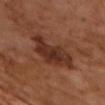Captured during whole-body skin photography for melanoma surveillance; the lesion was not biopsied.
The tile uses cross-polarized illumination.
The lesion's longest dimension is about 5.5 mm.
A close-up tile cropped from a whole-body skin photograph, about 15 mm across.
The lesion-visualizer software estimated an average lesion color of about L≈30 a*≈22 b*≈27 (CIELAB), roughly 10 lightness units darker than nearby skin, and a normalized border contrast of about 9.5. The analysis additionally found a within-lesion color-variation index near 4.5/10 and peripheral color asymmetry of about 1.5.
A male patient, roughly 65 years of age.